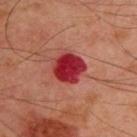Recorded during total-body skin imaging; not selected for excision or biopsy. From the upper back. A male patient approximately 50 years of age. The lesion-visualizer software estimated an eccentricity of roughly 0.3 and a symmetry-axis asymmetry near 0.15. It also reported a mean CIELAB color near L≈36 a*≈44 b*≈28 and a lesion–skin lightness drop of about 17. It also reported a border-irregularity rating of about 1.5/10 and peripheral color asymmetry of about 1. This is a cross-polarized tile. The recorded lesion diameter is about 3.5 mm. A 15 mm close-up tile from a total-body photography series done for melanoma screening.